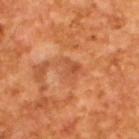Part of a total-body skin-imaging series; this lesion was reviewed on a skin check and was not flagged for biopsy.
Imaged with cross-polarized lighting.
A male subject approximately 65 years of age.
Automated image analysis of the tile measured a lesion area of about 5.5 mm², an eccentricity of roughly 0.55, and a shape-asymmetry score of about 0.4 (0 = symmetric). The analysis additionally found about 7 CIELAB-L* units darker than the surrounding skin and a normalized lesion–skin contrast near 5. The analysis additionally found a border-irregularity rating of about 4.5/10, a color-variation rating of about 4.5/10, and a peripheral color-asymmetry measure near 1.5. The analysis additionally found an automated nevus-likeness rating near 0 out of 100 and a detector confidence of about 100 out of 100 that the crop contains a lesion.
Measured at roughly 3 mm in maximum diameter.
A 15 mm close-up extracted from a 3D total-body photography capture.